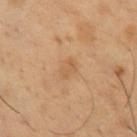Notes:
– biopsy status: total-body-photography surveillance lesion; no biopsy
– subject: male, aged 48 to 52
– lesion diameter: ~2.5 mm (longest diameter)
– location: the chest
– TBP lesion metrics: a classifier nevus-likeness of about 0/100
– image: 15 mm crop, total-body photography